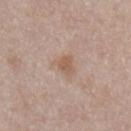Part of a total-body skin-imaging series; this lesion was reviewed on a skin check and was not flagged for biopsy. About 3 mm across. The subject is a male about 55 years old. A 15 mm close-up extracted from a 3D total-body photography capture. Automated image analysis of the tile measured a lesion color around L≈57 a*≈17 b*≈28 in CIELAB and a normalized lesion–skin contrast near 6.5. The analysis additionally found border irregularity of about 4 on a 0–10 scale, a within-lesion color-variation index near 2/10, and a peripheral color-asymmetry measure near 1. The lesion is located on the chest.